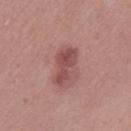Clinical impression:
The lesion was photographed on a routine skin check and not biopsied; there is no pathology result.
Image and clinical context:
A female subject, roughly 40 years of age. Cropped from a total-body skin-imaging series; the visible field is about 15 mm. From the right thigh. Longest diameter approximately 4.5 mm. The lesion-visualizer software estimated a border-irregularity rating of about 4.5/10, a color-variation rating of about 3/10, and peripheral color asymmetry of about 1. This is a white-light tile.